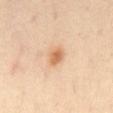<record>
  <biopsy_status>not biopsied; imaged during a skin examination</biopsy_status>
  <image>
    <source>total-body photography crop</source>
    <field_of_view_mm>15</field_of_view_mm>
  </image>
  <patient>
    <sex>male</sex>
    <age_approx>55</age_approx>
  </patient>
  <automated_metrics>
    <area_mm2_approx>3.5</area_mm2_approx>
    <eccentricity>0.65</eccentricity>
    <shape_asymmetry>0.25</shape_asymmetry>
    <cielab_L>67</cielab_L>
    <cielab_a>22</cielab_a>
    <cielab_b>39</cielab_b>
    <vs_skin_darker_L>11.0</vs_skin_darker_L>
    <vs_skin_contrast_norm>7.5</vs_skin_contrast_norm>
    <border_irregularity_0_10>2.0</border_irregularity_0_10>
    <color_variation_0_10>3.5</color_variation_0_10>
    <peripheral_color_asymmetry>1.0</peripheral_color_asymmetry>
    <nevus_likeness_0_100>90</nevus_likeness_0_100>
    <lesion_detection_confidence_0_100>100</lesion_detection_confidence_0_100>
  </automated_metrics>
  <site>abdomen</site>
  <lesion_size>
    <long_diameter_mm_approx>2.5</long_diameter_mm_approx>
  </lesion_size>
  <lighting>cross-polarized</lighting>
</record>